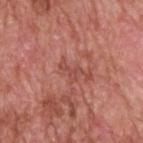The lesion was photographed on a routine skin check and not biopsied; there is no pathology result. A male patient roughly 60 years of age. Approximately 2.5 mm at its widest. This is a white-light tile. The lesion is located on the upper back. Cropped from a total-body skin-imaging series; the visible field is about 15 mm.